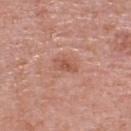{"patient": {"sex": "female", "age_approx": 60}, "image": {"source": "total-body photography crop", "field_of_view_mm": 15}, "site": "head or neck", "lesion_size": {"long_diameter_mm_approx": 2.5}, "lighting": "white-light"}